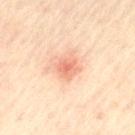No biopsy was performed on this lesion — it was imaged during a full skin examination and was not determined to be concerning. Captured under cross-polarized illumination. A 15 mm close-up extracted from a 3D total-body photography capture. Located on the left thigh. The subject is a male aged 58–62.